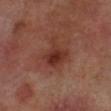The lesion was tiled from a total-body skin photograph and was not biopsied. On the right lower leg. Captured under cross-polarized illumination. Cropped from a total-body skin-imaging series; the visible field is about 15 mm. Approximately 4 mm at its widest. The subject is a male aged 68–72.